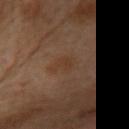biopsy status: total-body-photography surveillance lesion; no biopsy
image-analysis metrics: an area of roughly 5 mm² and a shape-asymmetry score of about 0.35 (0 = symmetric); a lesion color around L≈31 a*≈15 b*≈24 in CIELAB, about 4 CIELAB-L* units darker than the surrounding skin, and a lesion-to-skin contrast of about 5 (normalized; higher = more distinct); a peripheral color-asymmetry measure near 0.5; a classifier nevus-likeness of about 45/100
imaging modality: total-body-photography crop, ~15 mm field of view
subject: female, aged 58–62
size: ~3 mm (longest diameter)
site: the head or neck
tile lighting: cross-polarized